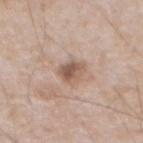– notes · catalogued during a skin exam; not biopsied
– patient · male, aged 63 to 67
– acquisition · ~15 mm crop, total-body skin-cancer survey
– size · ~3 mm (longest diameter)
– site · the abdomen
– tile lighting · white-light